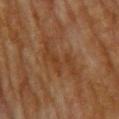workup: catalogued during a skin exam; not biopsied
lesion size: ~7.5 mm (longest diameter)
location: the upper back
imaging modality: total-body-photography crop, ~15 mm field of view
subject: female, aged 78–82